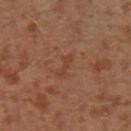Notes:
• biopsy status · catalogued during a skin exam; not biopsied
• location · the left lower leg
• lesion size · ~3 mm (longest diameter)
• lighting · cross-polarized illumination
• automated metrics · an area of roughly 2.5 mm² and a shape-asymmetry score of about 0.45 (0 = symmetric); a border-irregularity rating of about 5.5/10 and radial color variation of about 0; a classifier nevus-likeness of about 0/100 and a detector confidence of about 100 out of 100 that the crop contains a lesion
• imaging modality · ~15 mm crop, total-body skin-cancer survey
• subject · male, aged approximately 30